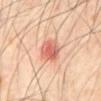Notes:
- notes · total-body-photography surveillance lesion; no biopsy
- image source · ~15 mm crop, total-body skin-cancer survey
- size · ≈3.5 mm
- body site · the mid back
- subject · male, aged around 60